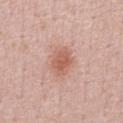Q: Automated lesion metrics?
A: a lesion-to-skin contrast of about 7 (normalized; higher = more distinct)
Q: What kind of image is this?
A: ~15 mm tile from a whole-body skin photo
Q: Lesion location?
A: the chest
Q: What are the patient's age and sex?
A: male, roughly 60 years of age